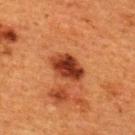notes: no biopsy performed (imaged during a skin exam); tile lighting: cross-polarized illumination; size: ~4.5 mm (longest diameter); image: ~15 mm tile from a whole-body skin photo; anatomic site: the upper back; patient: female, roughly 40 years of age.